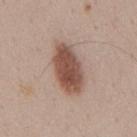The lesion is on the mid back.
Longest diameter approximately 6 mm.
A male subject, aged approximately 40.
Automated image analysis of the tile measured an outline eccentricity of about 0.85 (0 = round, 1 = elongated) and two-axis asymmetry of about 0.1. The analysis additionally found a border-irregularity index near 1.5/10, internal color variation of about 3.5 on a 0–10 scale, and a peripheral color-asymmetry measure near 1.5. It also reported an automated nevus-likeness rating near 100 out of 100.
A 15 mm crop from a total-body photograph taken for skin-cancer surveillance.
The tile uses white-light illumination.